An algorithmic analysis of the crop reported two-axis asymmetry of about 0.15. The analysis additionally found a border-irregularity rating of about 1.5/10, a color-variation rating of about 6/10, and radial color variation of about 1.5; a lesion tile, about 15 mm wide, cut from a 3D total-body photograph; imaged with white-light lighting; the patient is a male aged 58 to 62; from the upper back; the recorded lesion diameter is about 5 mm:
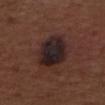Case summary:
– histopathology · a seborrheic keratosis — a benign skin lesion